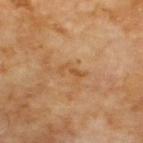follow-up — catalogued during a skin exam; not biopsied | location — the back | TBP lesion metrics — an outline eccentricity of about 0.9 (0 = round, 1 = elongated); a lesion–skin lightness drop of about 6; a border-irregularity rating of about 7/10, a within-lesion color-variation index near 0/10, and radial color variation of about 0 | patient — male, aged approximately 70 | acquisition — total-body-photography crop, ~15 mm field of view | size — ≈3 mm.